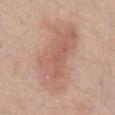Q: Is there a histopathology result?
A: imaged on a skin check; not biopsied
Q: What did automated image analysis measure?
A: an average lesion color of about L≈60 a*≈20 b*≈28 (CIELAB) and about 9 CIELAB-L* units darker than the surrounding skin; a border-irregularity rating of about 3.5/10, internal color variation of about 4 on a 0–10 scale, and a peripheral color-asymmetry measure near 1
Q: What kind of image is this?
A: 15 mm crop, total-body photography
Q: What is the lesion's diameter?
A: about 9 mm
Q: Where on the body is the lesion?
A: the abdomen
Q: Who is the patient?
A: male, roughly 55 years of age